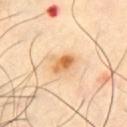workup: catalogued during a skin exam; not biopsied
diameter: about 3 mm
imaging modality: ~15 mm tile from a whole-body skin photo
patient: male, approximately 50 years of age
anatomic site: the chest